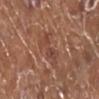An algorithmic analysis of the crop reported an area of roughly 6.5 mm². And it measured roughly 7 lightness units darker than nearby skin. It also reported a border-irregularity rating of about 5.5/10, a within-lesion color-variation index near 3/10, and radial color variation of about 1. Imaged with white-light lighting. The lesion is located on the leg. A roughly 15 mm field-of-view crop from a total-body skin photograph. A female patient in their mid- to late 70s. Approximately 4.5 mm at its widest.